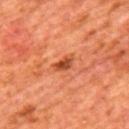biopsy status: total-body-photography surveillance lesion; no biopsy
lesion diameter: ≈3 mm
image: ~15 mm crop, total-body skin-cancer survey
patient: male, about 65 years old
location: the mid back